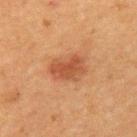Assessment: The lesion was photographed on a routine skin check and not biopsied; there is no pathology result. Context: This is a cross-polarized tile. Measured at roughly 4 mm in maximum diameter. This image is a 15 mm lesion crop taken from a total-body photograph. A male patient, approximately 50 years of age. On the left upper arm.